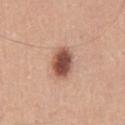anatomic site: the mid back; size: ≈3.5 mm; imaging modality: ~15 mm tile from a whole-body skin photo; subject: male, approximately 50 years of age; illumination: white-light illumination.